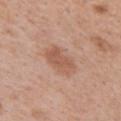{
  "biopsy_status": "not biopsied; imaged during a skin examination",
  "patient": {
    "sex": "female",
    "age_approx": 40
  },
  "site": "right upper arm",
  "automated_metrics": {
    "cielab_L": 56,
    "cielab_a": 22,
    "cielab_b": 30,
    "vs_skin_darker_L": 9.0,
    "vs_skin_contrast_norm": 6.0
  },
  "lesion_size": {
    "long_diameter_mm_approx": 4.5
  },
  "image": {
    "source": "total-body photography crop",
    "field_of_view_mm": 15
  },
  "lighting": "white-light"
}